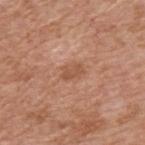The total-body-photography lesion software estimated an average lesion color of about L≈53 a*≈23 b*≈33 (CIELAB) and a normalized border contrast of about 6. The software also gave a border-irregularity index near 4/10, a within-lesion color-variation index near 1/10, and peripheral color asymmetry of about 0.5. And it measured a lesion-detection confidence of about 100/100. Longest diameter approximately 2.5 mm. A male patient, approximately 60 years of age. The lesion is on the back. Captured under white-light illumination. A region of skin cropped from a whole-body photographic capture, roughly 15 mm wide.